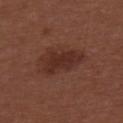{
  "biopsy_status": "not biopsied; imaged during a skin examination",
  "patient": {
    "sex": "male",
    "age_approx": 30
  },
  "image": {
    "source": "total-body photography crop",
    "field_of_view_mm": 15
  },
  "lesion_size": {
    "long_diameter_mm_approx": 5.0
  },
  "site": "upper back",
  "lighting": "white-light"
}